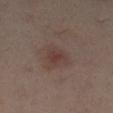This lesion was catalogued during total-body skin photography and was not selected for biopsy. A female patient, approximately 40 years of age. This is a cross-polarized tile. From the right leg. A 15 mm crop from a total-body photograph taken for skin-cancer surveillance.